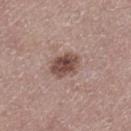  biopsy_status: not biopsied; imaged during a skin examination
  patient:
    sex: male
    age_approx: 70
  automated_metrics:
    cielab_L: 48
    cielab_a: 18
    cielab_b: 23
    vs_skin_contrast_norm: 9.5
    color_variation_0_10: 4.5
    peripheral_color_asymmetry: 1.5
  site: left thigh
  image:
    source: total-body photography crop
    field_of_view_mm: 15
  lighting: white-light